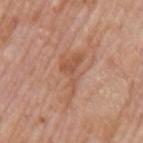workup = imaged on a skin check; not biopsied | site = the left upper arm | lesion size = ~6 mm (longest diameter) | patient = male, aged approximately 75 | image = ~15 mm crop, total-body skin-cancer survey | illumination = white-light.